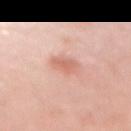The lesion is located on the right upper arm.
A female subject aged 43–47.
Approximately 2.5 mm at its widest.
Automated tile analysis of the lesion measured a classifier nevus-likeness of about 70/100.
A close-up tile cropped from a whole-body skin photograph, about 15 mm across.
This is a white-light tile.